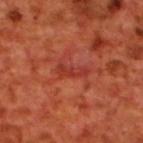Recorded during total-body skin imaging; not selected for excision or biopsy. On the upper back. The subject is a male in their 70s. An algorithmic analysis of the crop reported a lesion area of about 3.5 mm², an eccentricity of roughly 0.95, and a shape-asymmetry score of about 0.4 (0 = symmetric). It also reported a mean CIELAB color near L≈38 a*≈35 b*≈33, roughly 7 lightness units darker than nearby skin, and a lesion-to-skin contrast of about 5.5 (normalized; higher = more distinct). And it measured border irregularity of about 4 on a 0–10 scale, a color-variation rating of about 0/10, and a peripheral color-asymmetry measure near 0. And it measured an automated nevus-likeness rating near 0 out of 100. About 3.5 mm across. This is a cross-polarized tile. This image is a 15 mm lesion crop taken from a total-body photograph.